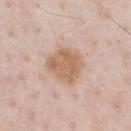Assessment:
Imaged during a routine full-body skin examination; the lesion was not biopsied and no histopathology is available.